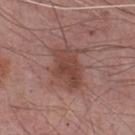| field | value |
|---|---|
| biopsy status | no biopsy performed (imaged during a skin exam) |
| location | the chest |
| image source | total-body-photography crop, ~15 mm field of view |
| illumination | white-light |
| diameter | about 5 mm |
| patient | male, in their mid- to late 70s |